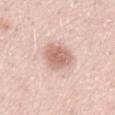The lesion was photographed on a routine skin check and not biopsied; there is no pathology result. From the left thigh. Cropped from a whole-body photographic skin survey; the tile spans about 15 mm. The subject is a male aged around 50. Approximately 4 mm at its widest.